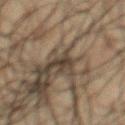* follow-up: no biopsy performed (imaged during a skin exam)
* image-analysis metrics: an outline eccentricity of about 0.9 (0 = round, 1 = elongated) and two-axis asymmetry of about 0.6; a within-lesion color-variation index near 0/10 and peripheral color asymmetry of about 0; a nevus-likeness score of about 0/100
* illumination: cross-polarized illumination
* imaging modality: total-body-photography crop, ~15 mm field of view
* patient: male, in their 60s
* size: ≈2 mm
* anatomic site: the chest